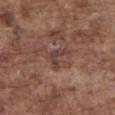Clinical impression:
The lesion was tiled from a total-body skin photograph and was not biopsied.
Clinical summary:
Longest diameter approximately 3 mm. A male patient, aged 73 to 77. A close-up tile cropped from a whole-body skin photograph, about 15 mm across. The tile uses white-light illumination. The lesion is located on the front of the torso.